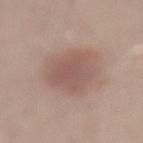notes = catalogued during a skin exam; not biopsied | image-analysis metrics = a lesion color around L≈54 a*≈18 b*≈24 in CIELAB, about 9 CIELAB-L* units darker than the surrounding skin, and a normalized lesion–skin contrast near 6.5; border irregularity of about 2.5 on a 0–10 scale, internal color variation of about 3 on a 0–10 scale, and radial color variation of about 1; a classifier nevus-likeness of about 75/100 and lesion-presence confidence of about 100/100 | image = total-body-photography crop, ~15 mm field of view | location = the lower back | tile lighting = white-light illumination | lesion size = about 6 mm | patient = male, aged approximately 30.